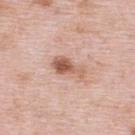Approximately 4 mm at its widest.
A female patient, in their mid-60s.
Cropped from a whole-body photographic skin survey; the tile spans about 15 mm.
This is a white-light tile.
From the back.